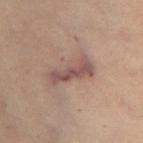Context: Cropped from a total-body skin-imaging series; the visible field is about 15 mm. The patient is a female about 60 years old. From the left thigh. The tile uses cross-polarized illumination.